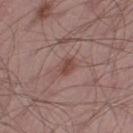Assessment:
This lesion was catalogued during total-body skin photography and was not selected for biopsy.
Background:
The lesion-visualizer software estimated a mean CIELAB color near L≈46 a*≈21 b*≈23 and a lesion-to-skin contrast of about 7 (normalized; higher = more distinct). And it measured a classifier nevus-likeness of about 45/100. About 3 mm across. A lesion tile, about 15 mm wide, cut from a 3D total-body photograph. A male subject aged 53–57. From the right thigh.